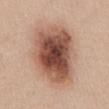follow-up: no biopsy performed (imaged during a skin exam)
size: ≈8 mm
image source: 15 mm crop, total-body photography
body site: the abdomen
subject: female, in their mid- to late 40s
automated metrics: a lesion area of about 41 mm², a shape eccentricity near 0.55, and a symmetry-axis asymmetry near 0.15; border irregularity of about 2 on a 0–10 scale and a color-variation rating of about 8.5/10; a nevus-likeness score of about 75/100 and lesion-presence confidence of about 100/100